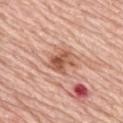notes: total-body-photography surveillance lesion; no biopsy
location: the upper back
subject: female, aged around 65
imaging modality: 15 mm crop, total-body photography